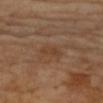site: the arm
acquisition: ~15 mm tile from a whole-body skin photo
subject: female, aged 68 to 72